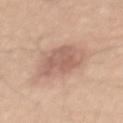* biopsy status · no biopsy performed (imaged during a skin exam)
* anatomic site · the mid back
* image-analysis metrics · an outline eccentricity of about 0.65 (0 = round, 1 = elongated); a mean CIELAB color near L≈60 a*≈20 b*≈26, roughly 10 lightness units darker than nearby skin, and a normalized lesion–skin contrast near 6.5; border irregularity of about 2 on a 0–10 scale, a within-lesion color-variation index near 3.5/10, and peripheral color asymmetry of about 1
* subject · male, in their mid-40s
* imaging modality · ~15 mm crop, total-body skin-cancer survey
* illumination · white-light